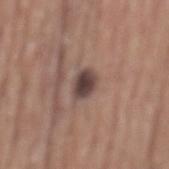Assessment: This lesion was catalogued during total-body skin photography and was not selected for biopsy. Clinical summary: The lesion's longest dimension is about 3 mm. This is a white-light tile. A male patient, roughly 75 years of age. Cropped from a total-body skin-imaging series; the visible field is about 15 mm. An algorithmic analysis of the crop reported a mean CIELAB color near L≈42 a*≈16 b*≈19 and about 14 CIELAB-L* units darker than the surrounding skin. The software also gave a detector confidence of about 100 out of 100 that the crop contains a lesion. The lesion is located on the mid back.